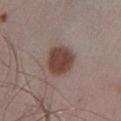Assessment: The lesion was tiled from a total-body skin photograph and was not biopsied. Context: The lesion is located on the left lower leg. A male patient roughly 30 years of age. Captured under white-light illumination. A 15 mm close-up tile from a total-body photography series done for melanoma screening. The recorded lesion diameter is about 4 mm.